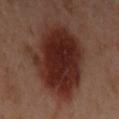A female patient roughly 40 years of age. Imaged with cross-polarized lighting. About 8.5 mm across. A region of skin cropped from a whole-body photographic capture, roughly 15 mm wide. The lesion is located on the left upper arm.